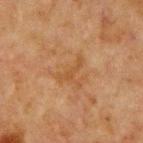| field | value |
|---|---|
| follow-up | imaged on a skin check; not biopsied |
| image-analysis metrics | a lesion area of about 2.5 mm² and a symmetry-axis asymmetry near 0.6; a classifier nevus-likeness of about 0/100 and a lesion-detection confidence of about 100/100 |
| acquisition | ~15 mm tile from a whole-body skin photo |
| patient | male, about 65 years old |
| illumination | cross-polarized |
| anatomic site | the chest |